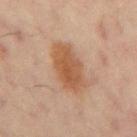* follow-up — imaged on a skin check; not biopsied
* illumination — cross-polarized illumination
* image-analysis metrics — a nevus-likeness score of about 95/100
* site — the mid back
* image source — total-body-photography crop, ~15 mm field of view
* subject — male, aged around 60
* lesion diameter — about 5.5 mm